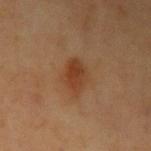Imaged during a routine full-body skin examination; the lesion was not biopsied and no histopathology is available.
The tile uses cross-polarized illumination.
Cropped from a whole-body photographic skin survey; the tile spans about 15 mm.
An algorithmic analysis of the crop reported an area of roughly 7 mm² and a shape eccentricity near 0.75. The analysis additionally found a classifier nevus-likeness of about 95/100 and lesion-presence confidence of about 100/100.
From the left upper arm.
A female subject, aged around 40.